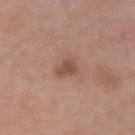Clinical impression:
The lesion was photographed on a routine skin check and not biopsied; there is no pathology result.
Clinical summary:
Measured at roughly 2.5 mm in maximum diameter. A male patient, aged 48 to 52. Cropped from a whole-body photographic skin survey; the tile spans about 15 mm. From the left upper arm. Automated image analysis of the tile measured a mean CIELAB color near L≈50 a*≈20 b*≈27, a lesion–skin lightness drop of about 9, and a lesion-to-skin contrast of about 6.5 (normalized; higher = more distinct). And it measured a border-irregularity rating of about 2.5/10, a color-variation rating of about 1.5/10, and a peripheral color-asymmetry measure near 0.5. Imaged with white-light lighting.